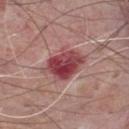Captured during whole-body skin photography for melanoma surveillance; the lesion was not biopsied. Located on the chest. Cropped from a total-body skin-imaging series; the visible field is about 15 mm. The patient is a male in their mid- to late 70s.